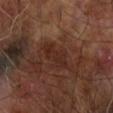Clinical impression: Recorded during total-body skin imaging; not selected for excision or biopsy. Image and clinical context: The lesion is on the right forearm. Approximately 4 mm at its widest. A 15 mm close-up tile from a total-body photography series done for melanoma screening. A male patient approximately 65 years of age.